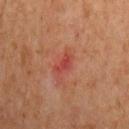biopsy_status: not biopsied; imaged during a skin examination